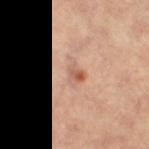<lesion>
<lighting>cross-polarized</lighting>
<automated_metrics>
  <cielab_L>57</cielab_L>
  <cielab_a>19</cielab_a>
  <cielab_b>28</cielab_b>
  <vs_skin_darker_L>7.0</vs_skin_darker_L>
  <nevus_likeness_0_100>30</nevus_likeness_0_100>
  <lesion_detection_confidence_0_100>100</lesion_detection_confidence_0_100>
</automated_metrics>
<patient>
  <sex>female</sex>
  <age_approx>65</age_approx>
</patient>
<image>
  <source>total-body photography crop</source>
  <field_of_view_mm>15</field_of_view_mm>
</image>
<lesion_size>
  <long_diameter_mm_approx>3.5</long_diameter_mm_approx>
</lesion_size>
<site>leg</site>
</lesion>